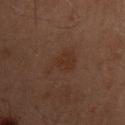biopsy status: no biopsy performed (imaged during a skin exam) | location: the mid back | acquisition: total-body-photography crop, ~15 mm field of view | patient: male, aged approximately 60.